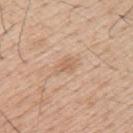Notes:
- biopsy status · total-body-photography surveillance lesion; no biopsy
- lesion diameter · ~2.5 mm (longest diameter)
- TBP lesion metrics · a lesion area of about 3.5 mm², an eccentricity of roughly 0.75, and a symmetry-axis asymmetry near 0.35; a classifier nevus-likeness of about 0/100 and lesion-presence confidence of about 100/100
- illumination · white-light
- site · the upper back
- image · total-body-photography crop, ~15 mm field of view
- subject · male, in their mid- to late 50s